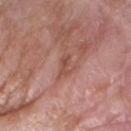Findings:
– biopsy status — total-body-photography surveillance lesion; no biopsy
– subject — male, aged around 60
– tile lighting — white-light
– lesion size — ~3 mm (longest diameter)
– anatomic site — the right forearm
– image source — total-body-photography crop, ~15 mm field of view